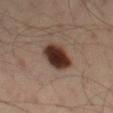biopsy status: catalogued during a skin exam; not biopsied | subject: male, aged 33–37 | location: the left thigh | imaging modality: 15 mm crop, total-body photography.